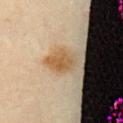Clinical impression:
The lesion was tiled from a total-body skin photograph and was not biopsied.
Acquisition and patient details:
The lesion's longest dimension is about 3.5 mm. The total-body-photography lesion software estimated an eccentricity of roughly 0.7 and two-axis asymmetry of about 0.2. And it measured border irregularity of about 2 on a 0–10 scale, internal color variation of about 4.5 on a 0–10 scale, and peripheral color asymmetry of about 1.5. On the abdomen. A female patient, in their mid-50s. Imaged with cross-polarized lighting. A close-up tile cropped from a whole-body skin photograph, about 15 mm across.